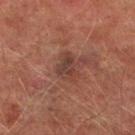The subject is a male in their mid- to late 70s. The lesion is located on the right lower leg. A 15 mm crop from a total-body photograph taken for skin-cancer surveillance.